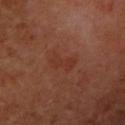No biopsy was performed on this lesion — it was imaged during a full skin examination and was not determined to be concerning.
A close-up tile cropped from a whole-body skin photograph, about 15 mm across.
The subject is a female aged 48–52.
The recorded lesion diameter is about 3.5 mm.
On the left forearm.